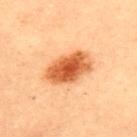Q: Was this lesion biopsied?
A: total-body-photography surveillance lesion; no biopsy
Q: Automated lesion metrics?
A: a mean CIELAB color near L≈61 a*≈31 b*≈45, a lesion–skin lightness drop of about 17, and a lesion-to-skin contrast of about 10.5 (normalized; higher = more distinct); a border-irregularity rating of about 1.5/10 and radial color variation of about 1.5
Q: What kind of image is this?
A: 15 mm crop, total-body photography
Q: Lesion location?
A: the upper back
Q: Lesion size?
A: ≈5.5 mm
Q: Who is the patient?
A: female, aged 33 to 37
Q: Illumination type?
A: cross-polarized